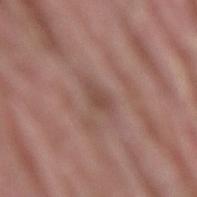Impression: No biopsy was performed on this lesion — it was imaged during a full skin examination and was not determined to be concerning. Clinical summary: The recorded lesion diameter is about 2.5 mm. This is a white-light tile. The lesion is located on the back. This image is a 15 mm lesion crop taken from a total-body photograph. The subject is a male approximately 40 years of age.